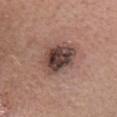Case summary:
* workup — no biopsy performed (imaged during a skin exam)